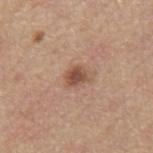Clinical impression: No biopsy was performed on this lesion — it was imaged during a full skin examination and was not determined to be concerning. Image and clinical context: The tile uses white-light illumination. A region of skin cropped from a whole-body photographic capture, roughly 15 mm wide. A male patient aged around 70. Automated tile analysis of the lesion measured a lesion color around L≈50 a*≈20 b*≈29 in CIELAB, roughly 12 lightness units darker than nearby skin, and a lesion-to-skin contrast of about 8.5 (normalized; higher = more distinct). And it measured a border-irregularity rating of about 2.5/10 and peripheral color asymmetry of about 0.5. The software also gave a classifier nevus-likeness of about 80/100 and a detector confidence of about 100 out of 100 that the crop contains a lesion. Approximately 2.5 mm at its widest. The lesion is located on the mid back.